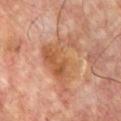| field | value |
|---|---|
| biopsy status | total-body-photography surveillance lesion; no biopsy |
| lesion diameter | ~7.5 mm (longest diameter) |
| lighting | cross-polarized |
| patient | male, about 55 years old |
| location | the front of the torso |
| imaging modality | total-body-photography crop, ~15 mm field of view |